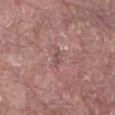{"biopsy_status": "not biopsied; imaged during a skin examination", "patient": {"sex": "male", "age_approx": 80}, "image": {"source": "total-body photography crop", "field_of_view_mm": 15}, "site": "abdomen"}